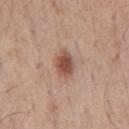Case summary:
* workup · no biopsy performed (imaged during a skin exam)
* tile lighting · white-light illumination
* imaging modality · ~15 mm tile from a whole-body skin photo
* site · the chest
* subject · male, approximately 55 years of age